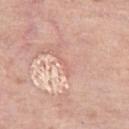Imaged during a routine full-body skin examination; the lesion was not biopsied and no histopathology is available. The lesion is located on the left thigh. A female patient aged 58–62. A 15 mm close-up extracted from a 3D total-body photography capture.